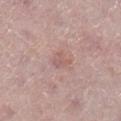{
  "biopsy_status": "not biopsied; imaged during a skin examination",
  "lighting": "white-light",
  "image": {
    "source": "total-body photography crop",
    "field_of_view_mm": 15
  },
  "lesion_size": {
    "long_diameter_mm_approx": 2.5
  },
  "patient": {
    "sex": "female",
    "age_approx": 50
  },
  "site": "right lower leg"
}